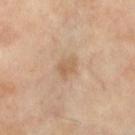Imaged during a routine full-body skin examination; the lesion was not biopsied and no histopathology is available. The tile uses cross-polarized illumination. The patient is a female aged 68–72. This image is a 15 mm lesion crop taken from a total-body photograph. The lesion is located on the left lower leg. The recorded lesion diameter is about 2.5 mm.